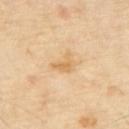Findings:
- workup — catalogued during a skin exam; not biopsied
- lesion diameter — ~2.5 mm (longest diameter)
- site — the back
- imaging modality — total-body-photography crop, ~15 mm field of view
- subject — male, aged 63 to 67
- lighting — cross-polarized